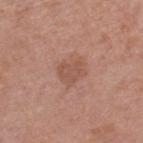Q: Was this lesion biopsied?
A: catalogued during a skin exam; not biopsied
Q: Who is the patient?
A: female, aged approximately 55
Q: What kind of image is this?
A: total-body-photography crop, ~15 mm field of view
Q: Where on the body is the lesion?
A: the right lower leg